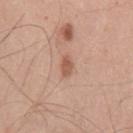biopsy status — catalogued during a skin exam; not biopsied
site — the front of the torso
imaging modality — 15 mm crop, total-body photography
automated lesion analysis — roughly 11 lightness units darker than nearby skin and a normalized lesion–skin contrast near 7.5; a border-irregularity rating of about 3/10, internal color variation of about 1 on a 0–10 scale, and radial color variation of about 0.5; lesion-presence confidence of about 100/100
patient — male, in their mid- to late 50s